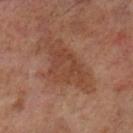Recorded during total-body skin imaging; not selected for excision or biopsy. The subject is a male aged 68–72. An algorithmic analysis of the crop reported a footprint of about 15 mm², an eccentricity of roughly 0.85, and a shape-asymmetry score of about 0.4 (0 = symmetric). And it measured border irregularity of about 5.5 on a 0–10 scale, a within-lesion color-variation index near 3/10, and peripheral color asymmetry of about 1. A roughly 15 mm field-of-view crop from a total-body skin photograph. From the left lower leg. The recorded lesion diameter is about 6.5 mm.